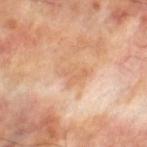Impression: The lesion was tiled from a total-body skin photograph and was not biopsied. Clinical summary: Cropped from a total-body skin-imaging series; the visible field is about 15 mm. On the left thigh. A male patient aged 68–72. The lesion's longest dimension is about 3.5 mm. Automated image analysis of the tile measured a nevus-likeness score of about 0/100.